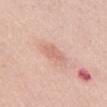Q: Was this lesion biopsied?
A: catalogued during a skin exam; not biopsied
Q: What kind of image is this?
A: 15 mm crop, total-body photography
Q: How was the tile lit?
A: white-light illumination
Q: What are the patient's age and sex?
A: male, approximately 55 years of age
Q: What is the lesion's diameter?
A: ~3 mm (longest diameter)
Q: Where on the body is the lesion?
A: the abdomen